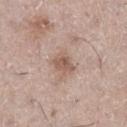Assessment:
Recorded during total-body skin imaging; not selected for excision or biopsy.
Image and clinical context:
The lesion-visualizer software estimated a classifier nevus-likeness of about 25/100 and lesion-presence confidence of about 100/100. A lesion tile, about 15 mm wide, cut from a 3D total-body photograph. On the leg. The patient is a female in their 60s. Approximately 3 mm at its widest. This is a white-light tile.